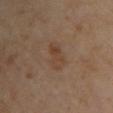The subject is a female about 45 years old. Approximately 3.5 mm at its widest. A roughly 15 mm field-of-view crop from a total-body skin photograph. The lesion is on the chest. The tile uses cross-polarized illumination.